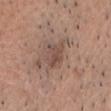workup = total-body-photography surveillance lesion; no biopsy
lesion size = about 2.5 mm
subject = male, about 40 years old
image = ~15 mm crop, total-body skin-cancer survey
anatomic site = the head or neck